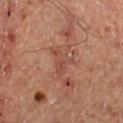{"biopsy_status": "not biopsied; imaged during a skin examination", "image": {"source": "total-body photography crop", "field_of_view_mm": 15}, "patient": {"sex": "male", "age_approx": 50}, "site": "right lower leg", "automated_metrics": {"eccentricity": 0.75, "shape_asymmetry": 0.6, "border_irregularity_0_10": 8.0, "color_variation_0_10": 1.0, "lesion_detection_confidence_0_100": 85}, "lesion_size": {"long_diameter_mm_approx": 3.5}, "lighting": "cross-polarized"}